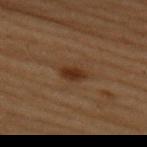Q: Was a biopsy performed?
A: no biopsy performed (imaged during a skin exam)
Q: What is the lesion's diameter?
A: ~3 mm (longest diameter)
Q: What are the patient's age and sex?
A: female, aged 48 to 52
Q: What is the anatomic site?
A: the upper back
Q: What did automated image analysis measure?
A: a border-irregularity rating of about 2/10, a color-variation rating of about 2/10, and peripheral color asymmetry of about 0.5; a nevus-likeness score of about 90/100 and a lesion-detection confidence of about 100/100
Q: What kind of image is this?
A: ~15 mm tile from a whole-body skin photo
Q: Illumination type?
A: cross-polarized illumination